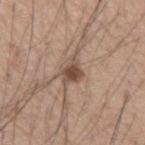Part of a total-body skin-imaging series; this lesion was reviewed on a skin check and was not flagged for biopsy.
Approximately 3 mm at its widest.
A 15 mm close-up tile from a total-body photography series done for melanoma screening.
The tile uses white-light illumination.
Located on the left forearm.
A male subject aged 58 to 62.